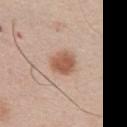Clinical impression:
Captured during whole-body skin photography for melanoma surveillance; the lesion was not biopsied.
Image and clinical context:
Approximately 3 mm at its widest. A male subject, aged 58 to 62. Cropped from a total-body skin-imaging series; the visible field is about 15 mm. On the chest.